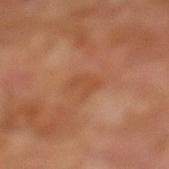Q: Was this lesion biopsied?
A: imaged on a skin check; not biopsied
Q: Who is the patient?
A: male, aged 68 to 72
Q: What is the imaging modality?
A: ~15 mm tile from a whole-body skin photo
Q: Lesion size?
A: ~2.5 mm (longest diameter)
Q: What is the anatomic site?
A: the leg